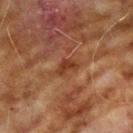biopsy_status: not biopsied; imaged during a skin examination
image:
  source: total-body photography crop
  field_of_view_mm: 15
patient:
  sex: male
  age_approx: 70
site: right upper arm
automated_metrics:
  area_mm2_approx: 3.5
  eccentricity: 0.8
  shape_asymmetry: 0.45
  border_irregularity_0_10: 4.5
  color_variation_0_10: 0.5
  peripheral_color_asymmetry: 0.0
  lesion_detection_confidence_0_100: 100
lesion_size:
  long_diameter_mm_approx: 2.5
lighting: cross-polarized